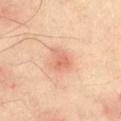Q: Is there a histopathology result?
A: no biopsy performed (imaged during a skin exam)
Q: Where on the body is the lesion?
A: the left thigh
Q: What is the imaging modality?
A: ~15 mm crop, total-body skin-cancer survey
Q: How was the tile lit?
A: cross-polarized
Q: What did automated image analysis measure?
A: a lesion area of about 6 mm², a shape eccentricity near 0.7, and a symmetry-axis asymmetry near 0.35; an average lesion color of about L≈69 a*≈27 b*≈33 (CIELAB), about 10 CIELAB-L* units darker than the surrounding skin, and a normalized lesion–skin contrast near 5.5; a border-irregularity rating of about 3/10, internal color variation of about 4 on a 0–10 scale, and radial color variation of about 1.5; a classifier nevus-likeness of about 5/100 and a detector confidence of about 100 out of 100 that the crop contains a lesion